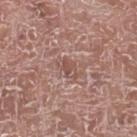| key | value |
|---|---|
| notes | no biopsy performed (imaged during a skin exam) |
| location | the left lower leg |
| patient | male, aged 73 to 77 |
| imaging modality | total-body-photography crop, ~15 mm field of view |
| automated metrics | an average lesion color of about L≈51 a*≈20 b*≈24 (CIELAB), about 8 CIELAB-L* units darker than the surrounding skin, and a normalized lesion–skin contrast near 5.5; a border-irregularity index near 3.5/10, internal color variation of about 1.5 on a 0–10 scale, and radial color variation of about 0.5 |
| lesion diameter | ≈2.5 mm |